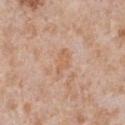The lesion was photographed on a routine skin check and not biopsied; there is no pathology result.
A 15 mm close-up tile from a total-body photography series done for melanoma screening.
The lesion is on the front of the torso.
Imaged with white-light lighting.
An algorithmic analysis of the crop reported an average lesion color of about L≈61 a*≈20 b*≈34 (CIELAB), roughly 6 lightness units darker than nearby skin, and a lesion-to-skin contrast of about 6 (normalized; higher = more distinct). It also reported internal color variation of about 0.5 on a 0–10 scale. It also reported a detector confidence of about 100 out of 100 that the crop contains a lesion.
A male subject aged around 65.
About 3.5 mm across.